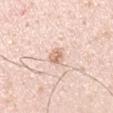| key | value |
|---|---|
| workup | total-body-photography surveillance lesion; no biopsy |
| patient | male, aged around 35 |
| imaging modality | 15 mm crop, total-body photography |
| location | the arm |